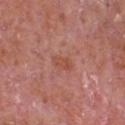follow-up = catalogued during a skin exam; not biopsied | lighting = white-light | imaging modality = 15 mm crop, total-body photography | subject = male, in their mid- to late 60s | lesion size = ~2.5 mm (longest diameter) | image-analysis metrics = an automated nevus-likeness rating near 0 out of 100 | body site = the chest.